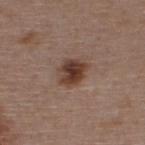Recorded during total-body skin imaging; not selected for excision or biopsy. Approximately 3.5 mm at its widest. Cropped from a whole-body photographic skin survey; the tile spans about 15 mm. On the upper back. A female subject, roughly 45 years of age. This is a white-light tile.